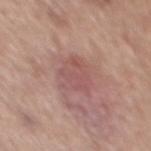No biopsy was performed on this lesion — it was imaged during a full skin examination and was not determined to be concerning. Approximately 4 mm at its widest. A close-up tile cropped from a whole-body skin photograph, about 15 mm across. An algorithmic analysis of the crop reported an area of roughly 11 mm², a shape eccentricity near 0.6, and a shape-asymmetry score of about 0.3 (0 = symmetric). It also reported an average lesion color of about L≈54 a*≈23 b*≈22 (CIELAB), about 7 CIELAB-L* units darker than the surrounding skin, and a normalized border contrast of about 5. The analysis additionally found a nevus-likeness score of about 0/100 and a detector confidence of about 100 out of 100 that the crop contains a lesion. The tile uses white-light illumination. A male patient aged approximately 70. On the mid back.